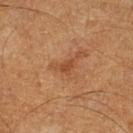The lesion is located on the left lower leg. A 15 mm close-up tile from a total-body photography series done for melanoma screening. Measured at roughly 2.5 mm in maximum diameter. The tile uses cross-polarized illumination. A male patient roughly 60 years of age.